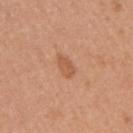<lesion>
<biopsy_status>not biopsied; imaged during a skin examination</biopsy_status>
<automated_metrics>
  <eccentricity>0.85</eccentricity>
  <shape_asymmetry>0.3</shape_asymmetry>
  <cielab_L>56</cielab_L>
  <cielab_a>24</cielab_a>
  <cielab_b>35</cielab_b>
  <vs_skin_darker_L>8.0</vs_skin_darker_L>
  <vs_skin_contrast_norm>6.0</vs_skin_contrast_norm>
  <border_irregularity_0_10>2.5</border_irregularity_0_10>
  <color_variation_0_10>2.0</color_variation_0_10>
  <peripheral_color_asymmetry>1.0</peripheral_color_asymmetry>
  <nevus_likeness_0_100>45</nevus_likeness_0_100>
  <lesion_detection_confidence_0_100>100</lesion_detection_confidence_0_100>
</automated_metrics>
<lighting>white-light</lighting>
<lesion_size>
  <long_diameter_mm_approx>3.0</long_diameter_mm_approx>
</lesion_size>
<patient>
  <sex>female</sex>
  <age_approx>35</age_approx>
</patient>
<site>right upper arm</site>
<image>
  <source>total-body photography crop</source>
  <field_of_view_mm>15</field_of_view_mm>
</image>
</lesion>